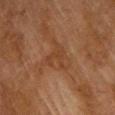Clinical impression: Imaged during a routine full-body skin examination; the lesion was not biopsied and no histopathology is available. Background: Cropped from a whole-body photographic skin survey; the tile spans about 15 mm. This is a cross-polarized tile. Longest diameter approximately 4 mm. The patient is a male aged 73–77. Located on the upper back.